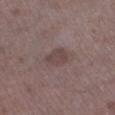Clinical impression:
Part of a total-body skin-imaging series; this lesion was reviewed on a skin check and was not flagged for biopsy.
Acquisition and patient details:
Captured under white-light illumination. A male patient aged around 70. This image is a 15 mm lesion crop taken from a total-body photograph. The total-body-photography lesion software estimated an area of roughly 5.5 mm², a shape eccentricity near 0.7, and a shape-asymmetry score of about 0.2 (0 = symmetric). The analysis additionally found a mean CIELAB color near L≈43 a*≈15 b*≈18, roughly 7 lightness units darker than nearby skin, and a lesion-to-skin contrast of about 6 (normalized; higher = more distinct). The analysis additionally found a nevus-likeness score of about 0/100 and a lesion-detection confidence of about 100/100. On the left lower leg.